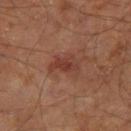Recorded during total-body skin imaging; not selected for excision or biopsy. Automated tile analysis of the lesion measured a lesion color around L≈37 a*≈23 b*≈26 in CIELAB, about 7 CIELAB-L* units darker than the surrounding skin, and a lesion-to-skin contrast of about 6.5 (normalized; higher = more distinct). And it measured border irregularity of about 4 on a 0–10 scale, internal color variation of about 3.5 on a 0–10 scale, and peripheral color asymmetry of about 1. And it measured a nevus-likeness score of about 15/100 and a lesion-detection confidence of about 100/100. Longest diameter approximately 3.5 mm. A male patient aged 58 to 62. Cropped from a whole-body photographic skin survey; the tile spans about 15 mm. Located on the right leg.